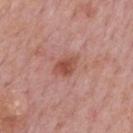biopsy status: no biopsy performed (imaged during a skin exam); patient: male, roughly 75 years of age; body site: the mid back; size: ~3 mm (longest diameter); image: total-body-photography crop, ~15 mm field of view.